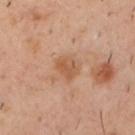{"biopsy_status": "not biopsied; imaged during a skin examination", "site": "upper back", "patient": {"sex": "male", "age_approx": 40}, "lesion_size": {"long_diameter_mm_approx": 3.0}, "image": {"source": "total-body photography crop", "field_of_view_mm": 15}, "automated_metrics": {"area_mm2_approx": 6.0, "eccentricity": 0.5, "shape_asymmetry": 0.15, "cielab_L": 54, "cielab_a": 21, "cielab_b": 33, "vs_skin_darker_L": 8.0, "vs_skin_contrast_norm": 6.0, "border_irregularity_0_10": 1.5, "peripheral_color_asymmetry": 1.0}, "lighting": "cross-polarized"}